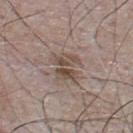<record>
  <biopsy_status>not biopsied; imaged during a skin examination</biopsy_status>
</record>